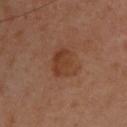workup: total-body-photography surveillance lesion; no biopsy | tile lighting: cross-polarized | lesion size: about 4 mm | subject: male, roughly 40 years of age | anatomic site: the upper back | acquisition: ~15 mm tile from a whole-body skin photo | TBP lesion metrics: an outline eccentricity of about 0.7 (0 = round, 1 = elongated) and a shape-asymmetry score of about 0.3 (0 = symmetric); a lesion color around L≈38 a*≈21 b*≈30 in CIELAB and a normalized lesion–skin contrast near 7.